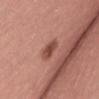The lesion was photographed on a routine skin check and not biopsied; there is no pathology result. Automated image analysis of the tile measured a footprint of about 4.5 mm² and an outline eccentricity of about 0.95 (0 = round, 1 = elongated). The software also gave a lesion–skin lightness drop of about 10 and a normalized lesion–skin contrast near 7.5. The analysis additionally found a border-irregularity rating of about 3/10, a color-variation rating of about 1.5/10, and a peripheral color-asymmetry measure near 0.5. Located on the back. Imaged with white-light lighting. A 15 mm close-up tile from a total-body photography series done for melanoma screening. About 3.5 mm across. A male subject, approximately 40 years of age.